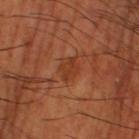follow-up: imaged on a skin check; not biopsied | site: the right thigh | tile lighting: cross-polarized illumination | image source: ~15 mm crop, total-body skin-cancer survey | image-analysis metrics: a border-irregularity index near 3/10, a color-variation rating of about 2.5/10, and peripheral color asymmetry of about 1 | lesion diameter: ~3 mm (longest diameter) | subject: male, aged approximately 65.